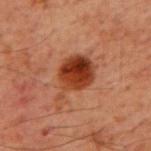No biopsy was performed on this lesion — it was imaged during a full skin examination and was not determined to be concerning.
A male patient aged approximately 60.
The recorded lesion diameter is about 5.5 mm.
Imaged with cross-polarized lighting.
A roughly 15 mm field-of-view crop from a total-body skin photograph.
The total-body-photography lesion software estimated a footprint of about 14 mm², a shape eccentricity near 0.75, and a symmetry-axis asymmetry near 0.35. It also reported a border-irregularity rating of about 4/10, a within-lesion color-variation index near 7/10, and a peripheral color-asymmetry measure near 2.5. The analysis additionally found lesion-presence confidence of about 100/100.
The lesion is located on the upper back.